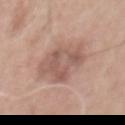Clinical impression: No biopsy was performed on this lesion — it was imaged during a full skin examination and was not determined to be concerning. Image and clinical context: A 15 mm crop from a total-body photograph taken for skin-cancer surveillance. From the chest. A male subject aged around 60.